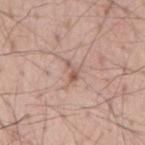The lesion was photographed on a routine skin check and not biopsied; there is no pathology result. The subject is a male aged approximately 55. The total-body-photography lesion software estimated a mean CIELAB color near L≈58 a*≈19 b*≈26 and about 9 CIELAB-L* units darker than the surrounding skin. The analysis additionally found a border-irregularity index near 4.5/10, a within-lesion color-variation index near 2.5/10, and radial color variation of about 0.5. Cropped from a whole-body photographic skin survey; the tile spans about 15 mm. Imaged with white-light lighting. Located on the mid back. Longest diameter approximately 3 mm.